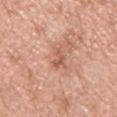Q: Was a biopsy performed?
A: imaged on a skin check; not biopsied
Q: What is the lesion's diameter?
A: ~2.5 mm (longest diameter)
Q: What is the imaging modality?
A: ~15 mm tile from a whole-body skin photo
Q: Lesion location?
A: the upper back
Q: Patient demographics?
A: male, approximately 55 years of age
Q: What lighting was used for the tile?
A: white-light illumination